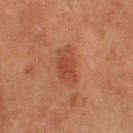The lesion was tiled from a total-body skin photograph and was not biopsied. A male patient aged approximately 60. The recorded lesion diameter is about 4.5 mm. This image is a 15 mm lesion crop taken from a total-body photograph. Automated image analysis of the tile measured an area of roughly 8 mm², an eccentricity of roughly 0.85, and a shape-asymmetry score of about 0.25 (0 = symmetric). And it measured a lesion color around L≈38 a*≈24 b*≈29 in CIELAB, roughly 7 lightness units darker than nearby skin, and a normalized lesion–skin contrast near 6.5. The software also gave a nevus-likeness score of about 20/100 and a detector confidence of about 100 out of 100 that the crop contains a lesion. The tile uses cross-polarized illumination. The lesion is on the upper back.